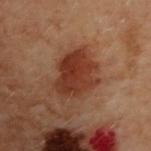* workup: catalogued during a skin exam; not biopsied
* acquisition: 15 mm crop, total-body photography
* location: the back
* patient: female, in their 60s